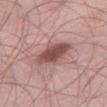Captured during whole-body skin photography for melanoma surveillance; the lesion was not biopsied. Captured under white-light illumination. The lesion is on the right thigh. Approximately 5 mm at its widest. A region of skin cropped from a whole-body photographic capture, roughly 15 mm wide. A male subject aged around 55. Automated tile analysis of the lesion measured a classifier nevus-likeness of about 65/100 and lesion-presence confidence of about 100/100.